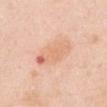The lesion was tiled from a total-body skin photograph and was not biopsied. A region of skin cropped from a whole-body photographic capture, roughly 15 mm wide. Located on the chest. The recorded lesion diameter is about 5.5 mm. A female patient, aged approximately 50. This is a white-light tile.